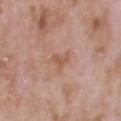Assessment:
Imaged during a routine full-body skin examination; the lesion was not biopsied and no histopathology is available.
Background:
Imaged with white-light lighting. A roughly 15 mm field-of-view crop from a total-body skin photograph. Approximately 3 mm at its widest. Automated tile analysis of the lesion measured an area of roughly 3.5 mm², an eccentricity of roughly 0.8, and a symmetry-axis asymmetry near 0.55. And it measured a mean CIELAB color near L≈56 a*≈21 b*≈31. The analysis additionally found a border-irregularity rating of about 5/10, a within-lesion color-variation index near 1.5/10, and radial color variation of about 0.5. The analysis additionally found a nevus-likeness score of about 0/100 and a lesion-detection confidence of about 100/100. The lesion is on the head or neck. A male patient, approximately 70 years of age.